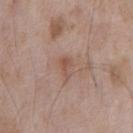Clinical summary:
A male subject aged approximately 65. Automated tile analysis of the lesion measured a lesion color around L≈52 a*≈19 b*≈27 in CIELAB, about 7 CIELAB-L* units darker than the surrounding skin, and a normalized lesion–skin contrast near 5.5. Cropped from a whole-body photographic skin survey; the tile spans about 15 mm. Located on the chest. The tile uses white-light illumination.